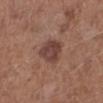Findings:
* workup — catalogued during a skin exam; not biopsied
* location — the left lower leg
* image — total-body-photography crop, ~15 mm field of view
* subject — male, aged 58 to 62